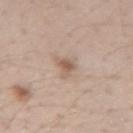No biopsy was performed on this lesion — it was imaged during a full skin examination and was not determined to be concerning. A female subject, roughly 40 years of age. A lesion tile, about 15 mm wide, cut from a 3D total-body photograph. The tile uses white-light illumination. The lesion is on the left forearm. Longest diameter approximately 2.5 mm.